A roughly 15 mm field-of-view crop from a total-body skin photograph. A female patient roughly 50 years of age. On the left thigh:
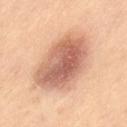Image and clinical context: Imaged with cross-polarized lighting. Automated tile analysis of the lesion measured a footprint of about 30 mm², a shape eccentricity near 0.75, and two-axis asymmetry of about 0.2. And it measured a lesion color around L≈61 a*≈23 b*≈30 in CIELAB and a lesion-to-skin contrast of about 9 (normalized; higher = more distinct). The software also gave a nevus-likeness score of about 90/100 and lesion-presence confidence of about 100/100. The recorded lesion diameter is about 7.5 mm. Conclusion: Histopathology of the biopsied lesion showed a benign skin lesion: dermatofibroma.